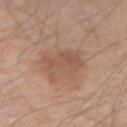No biopsy was performed on this lesion — it was imaged during a full skin examination and was not determined to be concerning.
A male patient, about 60 years old.
The lesion is on the left forearm.
A region of skin cropped from a whole-body photographic capture, roughly 15 mm wide.